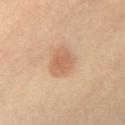Assessment: Captured during whole-body skin photography for melanoma surveillance; the lesion was not biopsied. Clinical summary: This image is a 15 mm lesion crop taken from a total-body photograph. Imaged with cross-polarized lighting. A female patient, aged 38 to 42. From the chest.